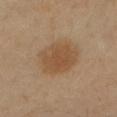Q: Is there a histopathology result?
A: no biopsy performed (imaged during a skin exam)
Q: How was this image acquired?
A: total-body-photography crop, ~15 mm field of view
Q: Where on the body is the lesion?
A: the left forearm
Q: Who is the patient?
A: female, approximately 40 years of age
Q: Lesion size?
A: ≈5 mm